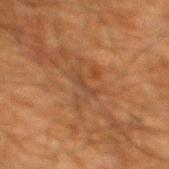From the mid back. The total-body-photography lesion software estimated a symmetry-axis asymmetry near 0.45. A male patient, aged around 60. A region of skin cropped from a whole-body photographic capture, roughly 15 mm wide. The lesion's longest dimension is about 2.5 mm.